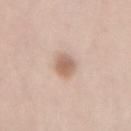• workup — no biopsy performed (imaged during a skin exam)
• size — about 3.5 mm
• patient — female, aged around 40
• tile lighting — white-light
• image-analysis metrics — a lesion area of about 5.5 mm², an eccentricity of roughly 0.85, and a symmetry-axis asymmetry near 0.2; a mean CIELAB color near L≈62 a*≈18 b*≈28 and roughly 12 lightness units darker than nearby skin; a border-irregularity rating of about 2/10 and radial color variation of about 1
• imaging modality — 15 mm crop, total-body photography
• site — the lower back